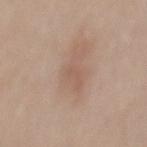Q: Was a biopsy performed?
A: catalogued during a skin exam; not biopsied
Q: How large is the lesion?
A: ~2 mm (longest diameter)
Q: Who is the patient?
A: male, aged 53 to 57
Q: How was the tile lit?
A: white-light
Q: Lesion location?
A: the mid back
Q: How was this image acquired?
A: ~15 mm tile from a whole-body skin photo
Q: Automated lesion metrics?
A: a mean CIELAB color near L≈55 a*≈19 b*≈27 and a lesion–skin lightness drop of about 5